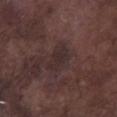The lesion was photographed on a routine skin check and not biopsied; there is no pathology result. The patient is a male in their mid- to late 70s. The lesion is on the left lower leg. A 15 mm crop from a total-body photograph taken for skin-cancer surveillance. Captured under white-light illumination.